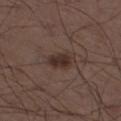{"biopsy_status": "not biopsied; imaged during a skin examination", "automated_metrics": {"area_mm2_approx": 6.5, "eccentricity": 0.8, "shape_asymmetry": 0.2, "cielab_L": 31, "cielab_a": 14, "cielab_b": 21, "vs_skin_contrast_norm": 8.5, "border_irregularity_0_10": 2.0, "color_variation_0_10": 2.5, "peripheral_color_asymmetry": 1.0, "nevus_likeness_0_100": 100}, "patient": {"sex": "male", "age_approx": 50}, "site": "left thigh", "image": {"source": "total-body photography crop", "field_of_view_mm": 15}, "lesion_size": {"long_diameter_mm_approx": 3.5}}